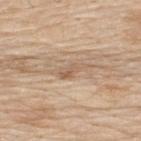Impression:
Imaged during a routine full-body skin examination; the lesion was not biopsied and no histopathology is available.
Clinical summary:
The tile uses white-light illumination. A male subject, roughly 80 years of age. A lesion tile, about 15 mm wide, cut from a 3D total-body photograph. Measured at roughly 3 mm in maximum diameter. The lesion is on the upper back.